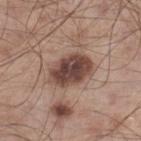{"biopsy_status": "not biopsied; imaged during a skin examination", "patient": {"sex": "male", "age_approx": 55}, "image": {"source": "total-body photography crop", "field_of_view_mm": 15}, "site": "right lower leg"}